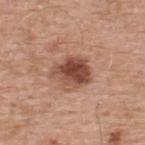The lesion was photographed on a routine skin check and not biopsied; there is no pathology result. Approximately 4.5 mm at its widest. A male patient aged approximately 55. A 15 mm crop from a total-body photograph taken for skin-cancer surveillance. The lesion is located on the upper back.